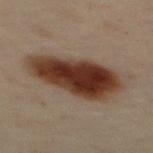– follow-up · catalogued during a skin exam; not biopsied
– location · the mid back
– acquisition · total-body-photography crop, ~15 mm field of view
– image-analysis metrics · an area of roughly 30 mm², a shape eccentricity near 0.85, and a shape-asymmetry score of about 0.25 (0 = symmetric); about 14 CIELAB-L* units darker than the surrounding skin; an automated nevus-likeness rating near 100 out of 100
– diameter · ≈8.5 mm
– illumination · cross-polarized illumination
– subject · male, roughly 50 years of age